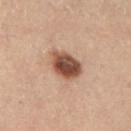Case summary:
* biopsy status: no biopsy performed (imaged during a skin exam)
* size: about 4 mm
* acquisition: ~15 mm tile from a whole-body skin photo
* illumination: cross-polarized
* patient: male, in their mid- to late 50s
* location: the left lower leg